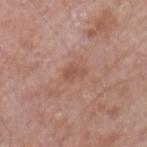workup: imaged on a skin check; not biopsied | tile lighting: white-light illumination | anatomic site: the arm | subject: male, aged 58–62 | image source: total-body-photography crop, ~15 mm field of view.